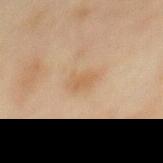Recorded during total-body skin imaging; not selected for excision or biopsy. A roughly 15 mm field-of-view crop from a total-body skin photograph. The patient is a male aged 43 to 47. From the mid back. Longest diameter approximately 3 mm. The tile uses cross-polarized illumination.